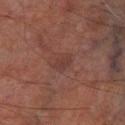- workup · total-body-photography surveillance lesion; no biopsy
- patient · male, approximately 70 years of age
- image · 15 mm crop, total-body photography
- anatomic site · the right lower leg